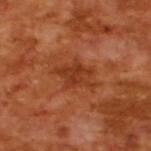Acquisition and patient details:
The patient is a male in their mid-60s. A region of skin cropped from a whole-body photographic capture, roughly 15 mm wide. The tile uses cross-polarized illumination.